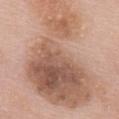Q: Was a biopsy performed?
A: catalogued during a skin exam; not biopsied
Q: Patient demographics?
A: female, aged 58 to 62
Q: Lesion size?
A: ~16 mm (longest diameter)
Q: Illumination type?
A: white-light illumination
Q: How was this image acquired?
A: total-body-photography crop, ~15 mm field of view
Q: Lesion location?
A: the mid back